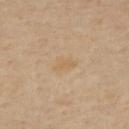Captured during whole-body skin photography for melanoma surveillance; the lesion was not biopsied. The patient is a female roughly 60 years of age. On the upper back. A 15 mm close-up tile from a total-body photography series done for melanoma screening. This is a cross-polarized tile. The total-body-photography lesion software estimated a border-irregularity index near 3/10, internal color variation of about 1.5 on a 0–10 scale, and radial color variation of about 0.5.